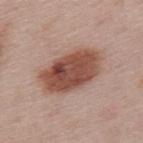On the upper back.
Automated image analysis of the tile measured two-axis asymmetry of about 0.15. It also reported an average lesion color of about L≈49 a*≈22 b*≈27 (CIELAB) and a normalized lesion–skin contrast near 10.5.
The subject is a female aged 48 to 52.
Approximately 7 mm at its widest.
A close-up tile cropped from a whole-body skin photograph, about 15 mm across.